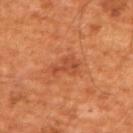Q: What kind of image is this?
A: ~15 mm tile from a whole-body skin photo
Q: Lesion size?
A: about 3.5 mm
Q: Patient demographics?
A: male, aged 63–67
Q: Lesion location?
A: the upper back
Q: Illumination type?
A: cross-polarized illumination
Q: What did automated image analysis measure?
A: roughly 8 lightness units darker than nearby skin and a normalized lesion–skin contrast near 6